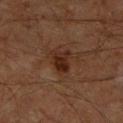notes: catalogued during a skin exam; not biopsied
size: ≈3 mm
tile lighting: cross-polarized
subject: male, aged 58 to 62
image source: 15 mm crop, total-body photography
TBP lesion metrics: an eccentricity of roughly 0.7 and two-axis asymmetry of about 0.35; an average lesion color of about L≈23 a*≈19 b*≈24 (CIELAB), roughly 9 lightness units darker than nearby skin, and a lesion-to-skin contrast of about 9.5 (normalized; higher = more distinct); an automated nevus-likeness rating near 65 out of 100 and a detector confidence of about 100 out of 100 that the crop contains a lesion
anatomic site: the right lower leg